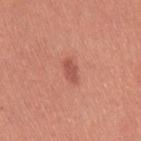notes — catalogued during a skin exam; not biopsied | automated lesion analysis — a footprint of about 3 mm² and two-axis asymmetry of about 0.3; a mean CIELAB color near L≈53 a*≈30 b*≈30 and a lesion-to-skin contrast of about 6.5 (normalized; higher = more distinct) | anatomic site — the left thigh | patient — female, aged approximately 35 | image source — 15 mm crop, total-body photography | tile lighting — white-light illumination.